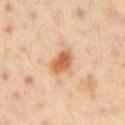Clinical impression: The lesion was tiled from a total-body skin photograph and was not biopsied. Clinical summary: From the arm. The subject is a male about 50 years old. The lesion's longest dimension is about 3.5 mm. A region of skin cropped from a whole-body photographic capture, roughly 15 mm wide. Captured under cross-polarized illumination.